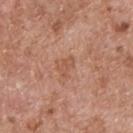<lesion>
<biopsy_status>not biopsied; imaged during a skin examination</biopsy_status>
<site>upper back</site>
<patient>
  <sex>male</sex>
  <age_approx>60</age_approx>
</patient>
<image>
  <source>total-body photography crop</source>
  <field_of_view_mm>15</field_of_view_mm>
</image>
</lesion>